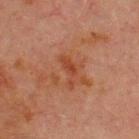• follow-up · total-body-photography surveillance lesion; no biopsy
• anatomic site · the chest
• lesion size · ~4 mm (longest diameter)
• image source · ~15 mm tile from a whole-body skin photo
• lighting · cross-polarized illumination
• subject · male, aged approximately 70